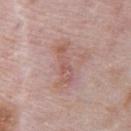Notes:
– follow-up — no biopsy performed (imaged during a skin exam)
– automated lesion analysis — a mean CIELAB color near L≈56 a*≈21 b*≈24, a lesion–skin lightness drop of about 8, and a normalized lesion–skin contrast near 5.5; a border-irregularity index near 4.5/10, a within-lesion color-variation index near 2.5/10, and a peripheral color-asymmetry measure near 0.5
– image source — 15 mm crop, total-body photography
– body site — the abdomen
– lesion size — ≈4.5 mm
– patient — male, aged around 70